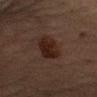- notes: catalogued during a skin exam; not biopsied
- patient: male, aged around 60
- anatomic site: the right upper arm
- tile lighting: cross-polarized illumination
- automated metrics: a footprint of about 9 mm², an outline eccentricity of about 0.85 (0 = round, 1 = elongated), and a symmetry-axis asymmetry near 0.2; a border-irregularity rating of about 2.5/10, a color-variation rating of about 3/10, and peripheral color asymmetry of about 1; an automated nevus-likeness rating near 95 out of 100
- acquisition: total-body-photography crop, ~15 mm field of view